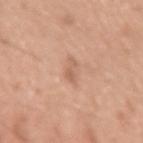biopsy_status: not biopsied; imaged during a skin examination
automated_metrics:
  area_mm2_approx: 2.5
  eccentricity: 0.95
  shape_asymmetry: 0.45
  cielab_L: 61
  cielab_a: 21
  cielab_b: 31
  vs_skin_darker_L: 8.0
  vs_skin_contrast_norm: 5.5
lesion_size:
  long_diameter_mm_approx: 3.0
site: left upper arm
image:
  source: total-body photography crop
  field_of_view_mm: 15
patient:
  sex: male
  age_approx: 20
lighting: white-light